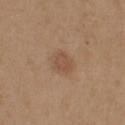Clinical impression:
Part of a total-body skin-imaging series; this lesion was reviewed on a skin check and was not flagged for biopsy.
Context:
Longest diameter approximately 3 mm. A roughly 15 mm field-of-view crop from a total-body skin photograph. A female subject, aged around 40. The tile uses white-light illumination. On the right upper arm.